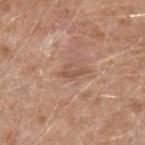Clinical impression:
The lesion was photographed on a routine skin check and not biopsied; there is no pathology result.
Background:
A lesion tile, about 15 mm wide, cut from a 3D total-body photograph. The lesion-visualizer software estimated a mean CIELAB color near L≈54 a*≈20 b*≈29 and roughly 8 lightness units darker than nearby skin. The software also gave a border-irregularity index near 5.5/10, internal color variation of about 0 on a 0–10 scale, and a peripheral color-asymmetry measure near 0. Imaged with white-light lighting. About 3 mm across. A male patient, aged approximately 60. Located on the right forearm.